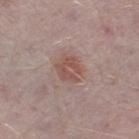Part of a total-body skin-imaging series; this lesion was reviewed on a skin check and was not flagged for biopsy.